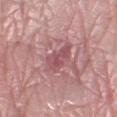- workup: no biopsy performed (imaged during a skin exam)